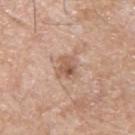follow-up — imaged on a skin check; not biopsied
image-analysis metrics — a lesion color around L≈57 a*≈20 b*≈29 in CIELAB, roughly 10 lightness units darker than nearby skin, and a normalized lesion–skin contrast near 7; a border-irregularity index near 4/10 and a within-lesion color-variation index near 6.5/10
anatomic site — the back
illumination — white-light
imaging modality — ~15 mm crop, total-body skin-cancer survey
subject — male, aged 53–57